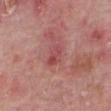Q: Is there a histopathology result?
A: imaged on a skin check; not biopsied
Q: How was this image acquired?
A: 15 mm crop, total-body photography
Q: Patient demographics?
A: male, aged 73–77
Q: What is the anatomic site?
A: the right forearm
Q: What is the lesion's diameter?
A: ~3 mm (longest diameter)
Q: How was the tile lit?
A: white-light
Q: Automated lesion metrics?
A: a shape eccentricity near 0.8; an average lesion color of about L≈49 a*≈31 b*≈23 (CIELAB), a lesion–skin lightness drop of about 8, and a lesion-to-skin contrast of about 6 (normalized; higher = more distinct); an automated nevus-likeness rating near 0 out of 100